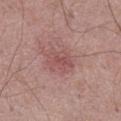follow-up = imaged on a skin check; not biopsied
patient = male, aged 68–72
anatomic site = the abdomen
imaging modality = 15 mm crop, total-body photography